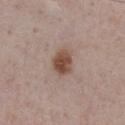Assessment:
Recorded during total-body skin imaging; not selected for excision or biopsy.
Acquisition and patient details:
About 3 mm across. A 15 mm close-up extracted from a 3D total-body photography capture. Located on the chest. The subject is a male aged around 55. The total-body-photography lesion software estimated border irregularity of about 2 on a 0–10 scale and internal color variation of about 3.5 on a 0–10 scale.